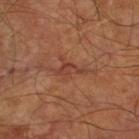notes: catalogued during a skin exam; not biopsied
image source: ~15 mm crop, total-body skin-cancer survey
patient: in their mid-60s
TBP lesion metrics: an area of roughly 3 mm² and two-axis asymmetry of about 0.6; an average lesion color of about L≈40 a*≈25 b*≈28 (CIELAB), a lesion–skin lightness drop of about 7, and a normalized lesion–skin contrast near 6.5; an automated nevus-likeness rating near 0 out of 100 and a lesion-detection confidence of about 80/100
anatomic site: the right thigh
diameter: ~3.5 mm (longest diameter)
lighting: cross-polarized illumination